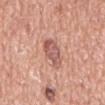biopsy status: catalogued during a skin exam; not biopsied | lesion size: ~4.5 mm (longest diameter) | anatomic site: the abdomen | tile lighting: white-light illumination | patient: male, roughly 75 years of age | imaging modality: 15 mm crop, total-body photography.